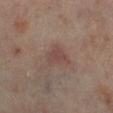Recorded during total-body skin imaging; not selected for excision or biopsy.